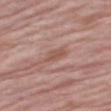The lesion was tiled from a total-body skin photograph and was not biopsied.